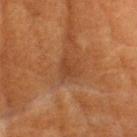| field | value |
|---|---|
| notes | total-body-photography surveillance lesion; no biopsy |
| lighting | cross-polarized |
| location | the head or neck |
| patient | female, roughly 80 years of age |
| diameter | about 2.5 mm |
| automated metrics | a footprint of about 4 mm², an outline eccentricity of about 0.6 (0 = round, 1 = elongated), and two-axis asymmetry of about 0.4; a lesion color around L≈31 a*≈19 b*≈28 in CIELAB, a lesion–skin lightness drop of about 5, and a normalized border contrast of about 5; border irregularity of about 3.5 on a 0–10 scale, internal color variation of about 1.5 on a 0–10 scale, and a peripheral color-asymmetry measure near 0.5 |
| acquisition | 15 mm crop, total-body photography |